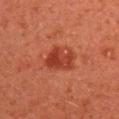notes: no biopsy performed (imaged during a skin exam) | image: ~15 mm tile from a whole-body skin photo | subject: male, about 45 years old | body site: the right upper arm.